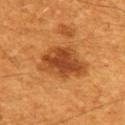follow-up: no biopsy performed (imaged during a skin exam)
patient: male, roughly 60 years of age
body site: the upper back
automated metrics: a footprint of about 18 mm², an eccentricity of roughly 0.8, and two-axis asymmetry of about 0.25; roughly 12 lightness units darker than nearby skin and a lesion-to-skin contrast of about 9 (normalized; higher = more distinct); a border-irregularity rating of about 3/10
lesion diameter: ≈6.5 mm
image source: 15 mm crop, total-body photography